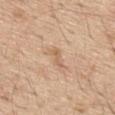Notes:
* workup — catalogued during a skin exam; not biopsied
* illumination — white-light
* location — the back
* acquisition — total-body-photography crop, ~15 mm field of view
* patient — male, roughly 70 years of age